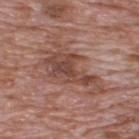| feature | finding |
|---|---|
| site | the upper back |
| imaging modality | ~15 mm crop, total-body skin-cancer survey |
| subject | male, aged approximately 70 |
| size | ~8 mm (longest diameter) |
| TBP lesion metrics | a mean CIELAB color near L≈46 a*≈21 b*≈25, a lesion–skin lightness drop of about 10, and a normalized border contrast of about 7.5 |
| illumination | white-light illumination |